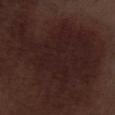Q: Was this lesion biopsied?
A: total-body-photography surveillance lesion; no biopsy
Q: How large is the lesion?
A: about 14 mm
Q: Patient demographics?
A: male, about 70 years old
Q: What kind of image is this?
A: ~15 mm crop, total-body skin-cancer survey
Q: What did automated image analysis measure?
A: an area of roughly 85 mm², a shape eccentricity near 0.7, and a symmetry-axis asymmetry near 0.55; an average lesion color of about L≈20 a*≈17 b*≈16 (CIELAB) and roughly 5 lightness units darker than nearby skin
Q: What is the anatomic site?
A: the left thigh
Q: Illumination type?
A: white-light